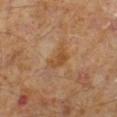follow-up: no biopsy performed (imaged during a skin exam) | size: ~3 mm (longest diameter) | image-analysis metrics: a lesion area of about 5 mm², a shape eccentricity near 0.8, and two-axis asymmetry of about 0.4; an average lesion color of about L≈45 a*≈19 b*≈35 (CIELAB) and roughly 7 lightness units darker than nearby skin; border irregularity of about 4 on a 0–10 scale, internal color variation of about 2 on a 0–10 scale, and a peripheral color-asymmetry measure near 0.5 | subject: male, aged approximately 60 | location: the left lower leg | imaging modality: 15 mm crop, total-body photography | tile lighting: cross-polarized illumination.